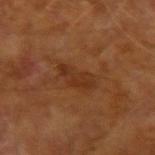This lesion was catalogued during total-body skin photography and was not selected for biopsy. A male patient aged 63–67. A 15 mm close-up tile from a total-body photography series done for melanoma screening. From the left forearm.